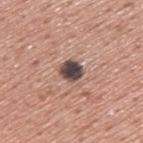Q: Was a biopsy performed?
A: no biopsy performed (imaged during a skin exam)
Q: How was this image acquired?
A: total-body-photography crop, ~15 mm field of view
Q: What is the anatomic site?
A: the upper back
Q: Patient demographics?
A: male, approximately 45 years of age